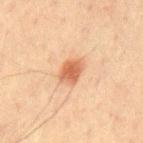Findings:
- follow-up · no biopsy performed (imaged during a skin exam)
- subject · male, approximately 70 years of age
- lighting · cross-polarized illumination
- anatomic site · the chest
- acquisition · ~15 mm crop, total-body skin-cancer survey
- TBP lesion metrics · a lesion area of about 6 mm² and two-axis asymmetry of about 0.2; a peripheral color-asymmetry measure near 0.5; an automated nevus-likeness rating near 100 out of 100 and a detector confidence of about 100 out of 100 that the crop contains a lesion
- lesion diameter · ~3 mm (longest diameter)